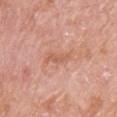subject = female, roughly 65 years of age; site = the upper back; image source = total-body-photography crop, ~15 mm field of view.